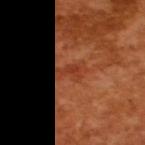patient: male, aged around 65
image source: ~15 mm crop, total-body skin-cancer survey
automated lesion analysis: an area of roughly 5 mm², an outline eccentricity of about 0.85 (0 = round, 1 = elongated), and a shape-asymmetry score of about 0.4 (0 = symmetric); a border-irregularity rating of about 4.5/10, a color-variation rating of about 2/10, and radial color variation of about 0.5
illumination: cross-polarized